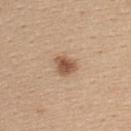follow-up — catalogued during a skin exam; not biopsied | size — ~3 mm (longest diameter) | subject — female, in their mid-40s | automated lesion analysis — a footprint of about 5 mm², an eccentricity of roughly 0.6, and a symmetry-axis asymmetry near 0.3; roughly 14 lightness units darker than nearby skin and a normalized border contrast of about 9; border irregularity of about 2.5 on a 0–10 scale, a color-variation rating of about 3.5/10, and peripheral color asymmetry of about 1; a classifier nevus-likeness of about 95/100 and a detector confidence of about 100 out of 100 that the crop contains a lesion | body site — the upper back | illumination — white-light | acquisition — total-body-photography crop, ~15 mm field of view.